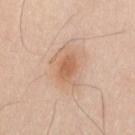{
  "biopsy_status": "not biopsied; imaged during a skin examination",
  "image": {
    "source": "total-body photography crop",
    "field_of_view_mm": 15
  },
  "site": "upper back",
  "patient": {
    "sex": "male",
    "age_approx": 35
  }
}